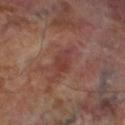Recorded during total-body skin imaging; not selected for excision or biopsy.
The subject is a male about 70 years old.
A lesion tile, about 15 mm wide, cut from a 3D total-body photograph.
On the left lower leg.